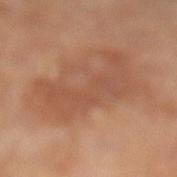Image and clinical context:
A 15 mm close-up tile from a total-body photography series done for melanoma screening. Approximately 7.5 mm at its widest. Captured under cross-polarized illumination. On the left lower leg. The patient is a male aged 63 to 67. The total-body-photography lesion software estimated a lesion color around L≈49 a*≈22 b*≈30 in CIELAB and about 6 CIELAB-L* units darker than the surrounding skin.